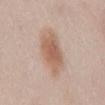Assessment:
Recorded during total-body skin imaging; not selected for excision or biopsy.
Clinical summary:
The subject is a male in their mid- to late 20s. The lesion is located on the mid back. A roughly 15 mm field-of-view crop from a total-body skin photograph. An algorithmic analysis of the crop reported a shape eccentricity near 0.9. It also reported a border-irregularity rating of about 2.5/10, a within-lesion color-variation index near 3.5/10, and a peripheral color-asymmetry measure near 1. The software also gave an automated nevus-likeness rating near 95 out of 100 and a detector confidence of about 100 out of 100 that the crop contains a lesion. This is a white-light tile.